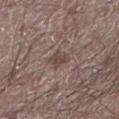Impression:
Captured during whole-body skin photography for melanoma surveillance; the lesion was not biopsied.
Clinical summary:
Approximately 2.5 mm at its widest. A region of skin cropped from a whole-body photographic capture, roughly 15 mm wide. The lesion is located on the leg. A male patient, aged approximately 65. An algorithmic analysis of the crop reported an average lesion color of about L≈44 a*≈15 b*≈21 (CIELAB) and about 8 CIELAB-L* units darker than the surrounding skin. The analysis additionally found an automated nevus-likeness rating near 0 out of 100 and a detector confidence of about 80 out of 100 that the crop contains a lesion.